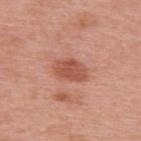Case summary:
- biopsy status · total-body-photography surveillance lesion; no biopsy
- image · 15 mm crop, total-body photography
- diameter · ~4 mm (longest diameter)
- patient · female, aged around 40
- anatomic site · the upper back